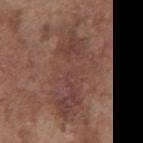Imaged with white-light lighting.
Automated image analysis of the tile measured roughly 7 lightness units darker than nearby skin.
The lesion is on the chest.
A region of skin cropped from a whole-body photographic capture, roughly 15 mm wide.
The lesion's longest dimension is about 11.5 mm.
A male patient aged 73–77.